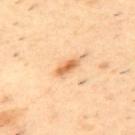Assessment:
Captured during whole-body skin photography for melanoma surveillance; the lesion was not biopsied.
Background:
A male subject, in their mid- to late 50s. This image is a 15 mm lesion crop taken from a total-body photograph. From the upper back.